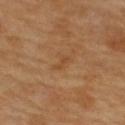The lesion was tiled from a total-body skin photograph and was not biopsied.
A female patient in their 60s.
The lesion is on the upper back.
Automated tile analysis of the lesion measured an average lesion color of about L≈48 a*≈21 b*≈37 (CIELAB) and a lesion-to-skin contrast of about 5 (normalized; higher = more distinct). The software also gave lesion-presence confidence of about 100/100.
A lesion tile, about 15 mm wide, cut from a 3D total-body photograph.